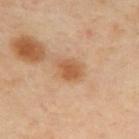workup — total-body-photography surveillance lesion; no biopsy | subject — female, aged around 40 | diameter — ~3 mm (longest diameter) | tile lighting — cross-polarized illumination | image source — ~15 mm crop, total-body skin-cancer survey | body site — the upper back.